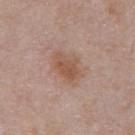The lesion was tiled from a total-body skin photograph and was not biopsied. A lesion tile, about 15 mm wide, cut from a 3D total-body photograph. A male subject aged approximately 55. Automated image analysis of the tile measured an area of roughly 8.5 mm² and a symmetry-axis asymmetry near 0.2. And it measured an average lesion color of about L≈54 a*≈19 b*≈29 (CIELAB), a lesion–skin lightness drop of about 8, and a normalized border contrast of about 7. The analysis additionally found a within-lesion color-variation index near 3.5/10. The software also gave a classifier nevus-likeness of about 45/100 and a detector confidence of about 100 out of 100 that the crop contains a lesion. Measured at roughly 4 mm in maximum diameter. The lesion is located on the chest. The tile uses white-light illumination.